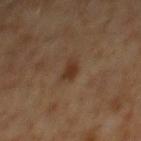Notes:
- biopsy status: catalogued during a skin exam; not biopsied
- lighting: cross-polarized
- body site: the mid back
- image source: 15 mm crop, total-body photography
- subject: male, in their 60s
- diameter: about 2.5 mm
- image-analysis metrics: a lesion area of about 3.5 mm²; an average lesion color of about L≈30 a*≈16 b*≈26 (CIELAB), about 8 CIELAB-L* units darker than the surrounding skin, and a normalized border contrast of about 8.5; a border-irregularity index near 3/10, a color-variation rating of about 1/10, and peripheral color asymmetry of about 0.5